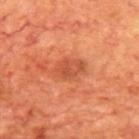<lesion>
<biopsy_status>not biopsied; imaged during a skin examination</biopsy_status>
<lesion_size>
  <long_diameter_mm_approx>3.5</long_diameter_mm_approx>
</lesion_size>
<lighting>cross-polarized</lighting>
<image>
  <source>total-body photography crop</source>
  <field_of_view_mm>15</field_of_view_mm>
</image>
<patient>
  <sex>male</sex>
  <age_approx>70</age_approx>
</patient>
<site>back</site>
</lesion>